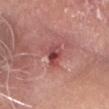location: the head or neck
size: ≈3 mm
image source: 15 mm crop, total-body photography
subject: male, approximately 65 years of age